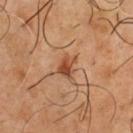– workup — imaged on a skin check; not biopsied
– anatomic site — the chest
– patient — male, aged approximately 50
– tile lighting — cross-polarized
– lesion size — ~2.5 mm (longest diameter)
– acquisition — ~15 mm tile from a whole-body skin photo